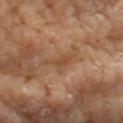Notes:
• workup — imaged on a skin check; not biopsied
• lesion size — about 3 mm
• acquisition — 15 mm crop, total-body photography
• tile lighting — cross-polarized illumination
• subject — male, aged 63–67